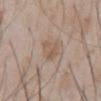Clinical impression:
No biopsy was performed on this lesion — it was imaged during a full skin examination and was not determined to be concerning.